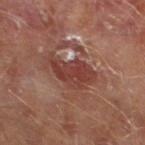Impression:
Part of a total-body skin-imaging series; this lesion was reviewed on a skin check and was not flagged for biopsy.
Context:
A roughly 15 mm field-of-view crop from a total-body skin photograph. The lesion is on the leg. Captured under cross-polarized illumination. Automated image analysis of the tile measured a lesion color around L≈39 a*≈25 b*≈25 in CIELAB and a lesion–skin lightness drop of about 9. And it measured a nevus-likeness score of about 0/100 and a lesion-detection confidence of about 95/100. The patient is a male about 65 years old. The lesion's longest dimension is about 5.5 mm.